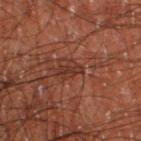follow-up = no biopsy performed (imaged during a skin exam) | image source = 15 mm crop, total-body photography | subject = male, aged 58–62 | body site = the right thigh.